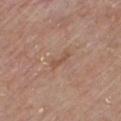Q: Is there a histopathology result?
A: no biopsy performed (imaged during a skin exam)
Q: What did automated image analysis measure?
A: roughly 7 lightness units darker than nearby skin and a normalized border contrast of about 6
Q: Where on the body is the lesion?
A: the chest
Q: Lesion size?
A: about 2.5 mm
Q: How was this image acquired?
A: total-body-photography crop, ~15 mm field of view
Q: Patient demographics?
A: male, aged approximately 80
Q: What lighting was used for the tile?
A: white-light illumination